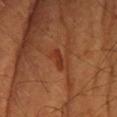workup: imaged on a skin check; not biopsied | image: ~15 mm crop, total-body skin-cancer survey | illumination: cross-polarized | anatomic site: the head or neck | diameter: ~3 mm (longest diameter) | patient: male, roughly 40 years of age.